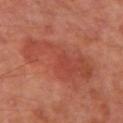A 15 mm crop from a total-body photograph taken for skin-cancer surveillance.
Automated image analysis of the tile measured an area of roughly 31 mm², an eccentricity of roughly 0.8, and a shape-asymmetry score of about 0.45 (0 = symmetric). The analysis additionally found an automated nevus-likeness rating near 50 out of 100 and lesion-presence confidence of about 100/100.
Located on the right upper arm.
A female patient, roughly 40 years of age.